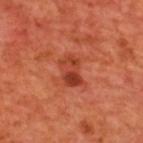- lesion size · ≈3.5 mm
- patient · male, aged 68 to 72
- location · the upper back
- image · ~15 mm tile from a whole-body skin photo
- tile lighting · cross-polarized illumination